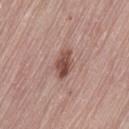Captured during whole-body skin photography for melanoma surveillance; the lesion was not biopsied. A female patient in their mid- to late 60s. Measured at roughly 3.5 mm in maximum diameter. On the lower back. An algorithmic analysis of the crop reported a footprint of about 6 mm², an outline eccentricity of about 0.8 (0 = round, 1 = elongated), and two-axis asymmetry of about 0.15. Cropped from a whole-body photographic skin survey; the tile spans about 15 mm. Captured under white-light illumination.